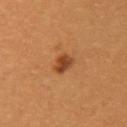The lesion was photographed on a routine skin check and not biopsied; there is no pathology result. This is a cross-polarized tile. A female subject roughly 30 years of age. The lesion is on the left upper arm. A roughly 15 mm field-of-view crop from a total-body skin photograph.